A 15 mm close-up tile from a total-body photography series done for melanoma screening, the lesion is located on the right thigh, a female subject aged 23–27.
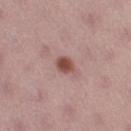This is a white-light tile.
Approximately 2 mm at its widest.
Histopathology of the biopsied lesion showed a dysplastic (Clark) nevus, classified as a benign lesion.